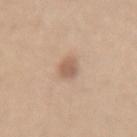Q: How was the tile lit?
A: white-light illumination
Q: What kind of image is this?
A: total-body-photography crop, ~15 mm field of view
Q: Patient demographics?
A: female, in their mid-50s
Q: Lesion location?
A: the mid back
Q: Lesion size?
A: ~3 mm (longest diameter)
Q: Automated lesion metrics?
A: an area of roughly 4 mm², a shape eccentricity near 0.8, and a shape-asymmetry score of about 0.2 (0 = symmetric); an average lesion color of about L≈59 a*≈18 b*≈29 (CIELAB)